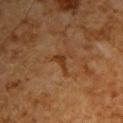Assessment: This lesion was catalogued during total-body skin photography and was not selected for biopsy. Background: On the upper back. The tile uses cross-polarized illumination. An algorithmic analysis of the crop reported an area of roughly 3 mm² and two-axis asymmetry of about 0.6. The software also gave an average lesion color of about L≈29 a*≈19 b*≈29 (CIELAB), roughly 6 lightness units darker than nearby skin, and a lesion-to-skin contrast of about 7 (normalized; higher = more distinct). The patient is a male roughly 65 years of age. A 15 mm close-up extracted from a 3D total-body photography capture. Longest diameter approximately 3 mm.